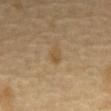<record>
<biopsy_status>not biopsied; imaged during a skin examination</biopsy_status>
<image>
  <source>total-body photography crop</source>
  <field_of_view_mm>15</field_of_view_mm>
</image>
<patient>
  <sex>male</sex>
  <age_approx>65</age_approx>
</patient>
<site>mid back</site>
</record>